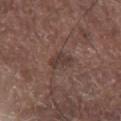notes: total-body-photography surveillance lesion; no biopsy | acquisition: total-body-photography crop, ~15 mm field of view | tile lighting: white-light | anatomic site: the chest | patient: male, aged approximately 80 | lesion diameter: about 3.5 mm.